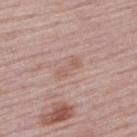Captured during whole-body skin photography for melanoma surveillance; the lesion was not biopsied. Measured at roughly 3.5 mm in maximum diameter. A female patient aged around 50. From the upper back. The lesion-visualizer software estimated border irregularity of about 4 on a 0–10 scale, a within-lesion color-variation index near 1/10, and a peripheral color-asymmetry measure near 0.5. The analysis additionally found a nevus-likeness score of about 0/100. A 15 mm close-up tile from a total-body photography series done for melanoma screening. This is a white-light tile.